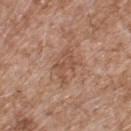The lesion was tiled from a total-body skin photograph and was not biopsied.
The total-body-photography lesion software estimated an eccentricity of roughly 0.7 and two-axis asymmetry of about 0.35. The analysis additionally found a mean CIELAB color near L≈52 a*≈20 b*≈30, a lesion–skin lightness drop of about 7, and a lesion-to-skin contrast of about 5.5 (normalized; higher = more distinct). The analysis additionally found a within-lesion color-variation index near 2.5/10 and a peripheral color-asymmetry measure near 1. It also reported an automated nevus-likeness rating near 0 out of 100.
Located on the back.
A close-up tile cropped from a whole-body skin photograph, about 15 mm across.
The patient is a male in their mid-50s.